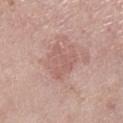- notes · catalogued during a skin exam; not biopsied
- body site · the left lower leg
- diameter · ≈4 mm
- subject · female, in their 70s
- tile lighting · white-light
- automated lesion analysis · an area of roughly 8.5 mm², an eccentricity of roughly 0.6, and two-axis asymmetry of about 0.5; a mean CIELAB color near L≈58 a*≈22 b*≈24, about 7 CIELAB-L* units darker than the surrounding skin, and a normalized border contrast of about 4.5
- image source · ~15 mm tile from a whole-body skin photo